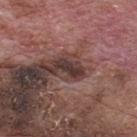Part of a total-body skin-imaging series; this lesion was reviewed on a skin check and was not flagged for biopsy.
A male patient, in their 70s.
The lesion is located on the back.
A 15 mm crop from a total-body photograph taken for skin-cancer surveillance.